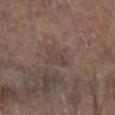biopsy status = total-body-photography surveillance lesion; no biopsy
imaging modality = ~15 mm tile from a whole-body skin photo
subject = female, aged approximately 85 or older
site = the left forearm
tile lighting = white-light illumination
size = ≈3 mm